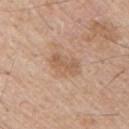{"biopsy_status": "not biopsied; imaged during a skin examination"}